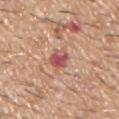Imaged during a routine full-body skin examination; the lesion was not biopsied and no histopathology is available.
A male subject, in their 60s.
Cropped from a whole-body photographic skin survey; the tile spans about 15 mm.
The lesion is located on the chest.